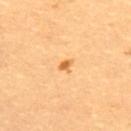Impression: Captured during whole-body skin photography for melanoma surveillance; the lesion was not biopsied. Acquisition and patient details: Captured under cross-polarized illumination. The subject is a female aged around 30. Located on the upper back. The lesion's longest dimension is about 2 mm. Cropped from a whole-body photographic skin survey; the tile spans about 15 mm.